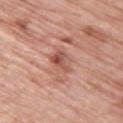Q: Was this lesion biopsied?
A: catalogued during a skin exam; not biopsied
Q: Lesion location?
A: the upper back
Q: How was the tile lit?
A: white-light illumination
Q: How was this image acquired?
A: ~15 mm crop, total-body skin-cancer survey
Q: What are the patient's age and sex?
A: female, in their mid- to late 60s